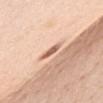Impression:
This lesion was catalogued during total-body skin photography and was not selected for biopsy.
Acquisition and patient details:
Longest diameter approximately 3 mm. The subject is a female aged approximately 45. Automated tile analysis of the lesion measured a lesion color around L≈63 a*≈23 b*≈30 in CIELAB and a lesion–skin lightness drop of about 15. The software also gave a border-irregularity rating of about 3/10, a within-lesion color-variation index near 0/10, and a peripheral color-asymmetry measure near 0. The tile uses white-light illumination. From the chest. A 15 mm close-up extracted from a 3D total-body photography capture.